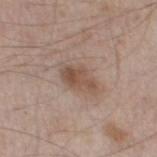Q: Is there a histopathology result?
A: total-body-photography surveillance lesion; no biopsy
Q: What is the imaging modality?
A: total-body-photography crop, ~15 mm field of view
Q: Illumination type?
A: white-light illumination
Q: Who is the patient?
A: male, aged 53–57
Q: What is the anatomic site?
A: the left thigh